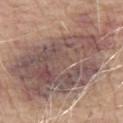Q: Is there a histopathology result?
A: catalogued during a skin exam; not biopsied
Q: Illumination type?
A: white-light
Q: Lesion location?
A: the arm
Q: Patient demographics?
A: male, in their mid- to late 60s
Q: Lesion size?
A: ~14.5 mm (longest diameter)
Q: What is the imaging modality?
A: ~15 mm tile from a whole-body skin photo
Q: Automated lesion metrics?
A: an area of roughly 85 mm², an eccentricity of roughly 0.8, and a shape-asymmetry score of about 0.3 (0 = symmetric)